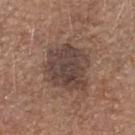Assessment:
No biopsy was performed on this lesion — it was imaged during a full skin examination and was not determined to be concerning.
Acquisition and patient details:
The recorded lesion diameter is about 5.5 mm. The patient is a female aged 73 to 77. On the head or neck. Automated image analysis of the tile measured an average lesion color of about L≈42 a*≈16 b*≈21 (CIELAB), about 10 CIELAB-L* units darker than the surrounding skin, and a normalized lesion–skin contrast near 9. Imaged with white-light lighting. A 15 mm crop from a total-body photograph taken for skin-cancer surveillance.